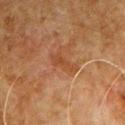Q: Was a biopsy performed?
A: total-body-photography surveillance lesion; no biopsy
Q: Who is the patient?
A: male, aged approximately 80
Q: Automated lesion metrics?
A: a footprint of about 4.5 mm², an eccentricity of roughly 0.9, and two-axis asymmetry of about 0.4; a mean CIELAB color near L≈36 a*≈20 b*≈30, about 5 CIELAB-L* units darker than the surrounding skin, and a normalized border contrast of about 5.5; a border-irregularity index near 4.5/10, internal color variation of about 1 on a 0–10 scale, and peripheral color asymmetry of about 0; a nevus-likeness score of about 0/100 and lesion-presence confidence of about 100/100
Q: What is the lesion's diameter?
A: ~3.5 mm (longest diameter)
Q: Where on the body is the lesion?
A: the arm
Q: How was this image acquired?
A: ~15 mm tile from a whole-body skin photo